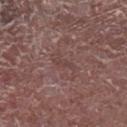Captured during whole-body skin photography for melanoma surveillance; the lesion was not biopsied.
From the leg.
Imaged with white-light lighting.
The lesion's longest dimension is about 2.5 mm.
A male patient aged 63–67.
A region of skin cropped from a whole-body photographic capture, roughly 15 mm wide.